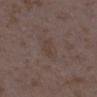Findings:
– workup: total-body-photography surveillance lesion; no biopsy
– illumination: white-light illumination
– image source: 15 mm crop, total-body photography
– site: the right lower leg
– subject: female, in their mid- to late 30s
– automated lesion analysis: a border-irregularity rating of about 3/10, a color-variation rating of about 0/10, and peripheral color asymmetry of about 0